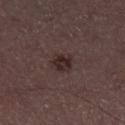This lesion was catalogued during total-body skin photography and was not selected for biopsy.
Captured under white-light illumination.
A 15 mm crop from a total-body photograph taken for skin-cancer surveillance.
A male subject about 55 years old.
From the right lower leg.